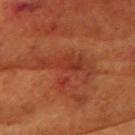{"biopsy_status": "not biopsied; imaged during a skin examination", "patient": {"sex": "female", "age_approx": 80}, "site": "head or neck", "image": {"source": "total-body photography crop", "field_of_view_mm": 15}, "lesion_size": {"long_diameter_mm_approx": 6.5}}